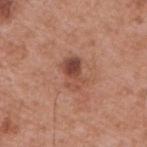Impression: The lesion was tiled from a total-body skin photograph and was not biopsied. Background: A male subject aged approximately 55. About 3.5 mm across. From the upper back. This is a white-light tile. Cropped from a total-body skin-imaging series; the visible field is about 15 mm.